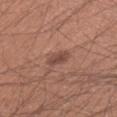Assessment: Captured during whole-body skin photography for melanoma surveillance; the lesion was not biopsied. Image and clinical context: Captured under white-light illumination. The patient is a male in their mid-30s. On the arm. Cropped from a total-body skin-imaging series; the visible field is about 15 mm.